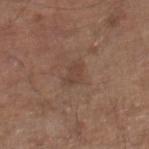  biopsy_status: not biopsied; imaged during a skin examination
  lesion_size:
    long_diameter_mm_approx: 3.0
  site: leg
  lighting: white-light
  image:
    source: total-body photography crop
    field_of_view_mm: 15
  patient:
    sex: male
    age_approx: 80
  automated_metrics:
    eccentricity: 0.75
    shape_asymmetry: 0.35
    border_irregularity_0_10: 3.5
    color_variation_0_10: 1.5